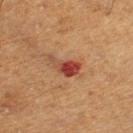| field | value |
|---|---|
| workup | imaged on a skin check; not biopsied |
| automated lesion analysis | a lesion area of about 5.5 mm² and a symmetry-axis asymmetry near 0.45; a lesion color around L≈39 a*≈27 b*≈29 in CIELAB, about 12 CIELAB-L* units darker than the surrounding skin, and a normalized border contrast of about 10 |
| anatomic site | the right thigh |
| imaging modality | ~15 mm crop, total-body skin-cancer survey |
| lesion diameter | ~3.5 mm (longest diameter) |
| lighting | cross-polarized |
| subject | male, in their mid-50s |